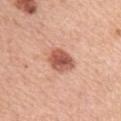Background: A 15 mm close-up extracted from a 3D total-body photography capture. The lesion is on the left upper arm. Automated tile analysis of the lesion measured an automated nevus-likeness rating near 95 out of 100. A female patient, approximately 60 years of age.